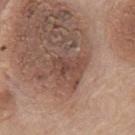From the chest. A male patient, approximately 75 years of age. A region of skin cropped from a whole-body photographic capture, roughly 15 mm wide. Imaged with white-light lighting. The recorded lesion diameter is about 4 mm.